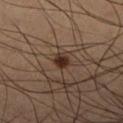Impression: Recorded during total-body skin imaging; not selected for excision or biopsy. Image and clinical context: A 15 mm close-up extracted from a 3D total-body photography capture. The lesion is on the leg. The recorded lesion diameter is about 2 mm. Automated image analysis of the tile measured a lesion area of about 3 mm², an eccentricity of roughly 0.55, and a symmetry-axis asymmetry near 0.25. It also reported an average lesion color of about L≈28 a*≈17 b*≈23 (CIELAB) and a lesion–skin lightness drop of about 13. The analysis additionally found a border-irregularity index near 2/10, a color-variation rating of about 1/10, and peripheral color asymmetry of about 0.5. A male subject aged 63–67. This is a cross-polarized tile.